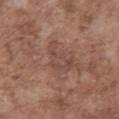biopsy status — catalogued during a skin exam; not biopsied
image — ~15 mm crop, total-body skin-cancer survey
image-analysis metrics — an eccentricity of roughly 0.85; a lesion color around L≈46 a*≈20 b*≈24 in CIELAB, roughly 6 lightness units darker than nearby skin, and a lesion-to-skin contrast of about 5 (normalized; higher = more distinct)
subject — male, about 75 years old
lighting — white-light
location — the abdomen